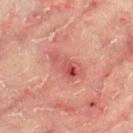biopsy_status: not biopsied; imaged during a skin examination
patient:
  sex: male
  age_approx: 65
image:
  source: total-body photography crop
  field_of_view_mm: 15
automated_metrics:
  cielab_L: 49
  cielab_a: 29
  cielab_b: 25
  vs_skin_contrast_norm: 6.5
  color_variation_0_10: 9.5
  peripheral_color_asymmetry: 3.5
  nevus_likeness_0_100: 0
  lesion_detection_confidence_0_100: 100
site: left thigh